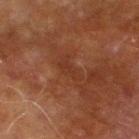Part of a total-body skin-imaging series; this lesion was reviewed on a skin check and was not flagged for biopsy.
The recorded lesion diameter is about 3.5 mm.
Automated image analysis of the tile measured a border-irregularity rating of about 5/10 and radial color variation of about 0. It also reported a classifier nevus-likeness of about 0/100 and a detector confidence of about 75 out of 100 that the crop contains a lesion.
A male subject, roughly 70 years of age.
Located on the right lower leg.
Captured under cross-polarized illumination.
This image is a 15 mm lesion crop taken from a total-body photograph.